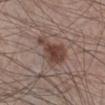Clinical summary: A lesion tile, about 15 mm wide, cut from a 3D total-body photograph. The patient is a male aged 58–62. Captured under white-light illumination. Measured at roughly 5 mm in maximum diameter. Located on the right lower leg.